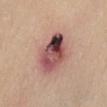This lesion was catalogued during total-body skin photography and was not selected for biopsy. The recorded lesion diameter is about 6 mm. Captured under white-light illumination. The lesion is located on the abdomen. The patient is a female approximately 40 years of age. A 15 mm crop from a total-body photograph taken for skin-cancer surveillance.